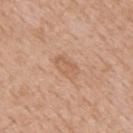Impression:
Captured during whole-body skin photography for melanoma surveillance; the lesion was not biopsied.
Acquisition and patient details:
Approximately 3.5 mm at its widest. A close-up tile cropped from a whole-body skin photograph, about 15 mm across. From the mid back. The tile uses white-light illumination. The total-body-photography lesion software estimated a nevus-likeness score of about 0/100. A male patient, in their mid-60s.